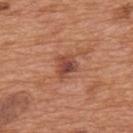Recorded during total-body skin imaging; not selected for excision or biopsy.
Cropped from a whole-body photographic skin survey; the tile spans about 15 mm.
The lesion is located on the back.
Automated tile analysis of the lesion measured a lesion color around L≈46 a*≈26 b*≈30 in CIELAB, a lesion–skin lightness drop of about 11, and a normalized lesion–skin contrast near 8.
Measured at roughly 2.5 mm in maximum diameter.
This is a white-light tile.
The subject is a male in their mid-60s.